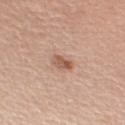This lesion was catalogued during total-body skin photography and was not selected for biopsy. A female patient aged approximately 45. The recorded lesion diameter is about 2.5 mm. Cropped from a total-body skin-imaging series; the visible field is about 15 mm. Automated tile analysis of the lesion measured an area of roughly 4 mm² and an outline eccentricity of about 0.85 (0 = round, 1 = elongated). The analysis additionally found a border-irregularity rating of about 2.5/10, a color-variation rating of about 3/10, and a peripheral color-asymmetry measure near 1. The software also gave a classifier nevus-likeness of about 35/100. The lesion is located on the right upper arm. The tile uses white-light illumination.